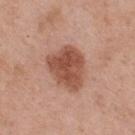Impression:
The lesion was photographed on a routine skin check and not biopsied; there is no pathology result.
Acquisition and patient details:
Automated image analysis of the tile measured a lesion color around L≈51 a*≈24 b*≈30 in CIELAB, a lesion–skin lightness drop of about 13, and a normalized border contrast of about 9. And it measured a border-irregularity rating of about 3/10 and a within-lesion color-variation index near 3.5/10. It also reported an automated nevus-likeness rating near 85 out of 100. Approximately 5 mm at its widest. Captured under white-light illumination. On the upper back. The subject is a male roughly 55 years of age. This image is a 15 mm lesion crop taken from a total-body photograph.